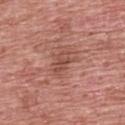Recorded during total-body skin imaging; not selected for excision or biopsy. This image is a 15 mm lesion crop taken from a total-body photograph. A male subject roughly 60 years of age. The lesion is on the back. Imaged with white-light lighting. Approximately 3.5 mm at its widest. The lesion-visualizer software estimated an area of roughly 4.5 mm², a shape eccentricity near 0.8, and a symmetry-axis asymmetry near 0.35.